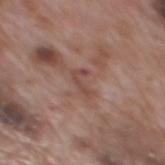Clinical impression: The lesion was photographed on a routine skin check and not biopsied; there is no pathology result. Clinical summary: The lesion is on the mid back. A male patient aged around 70. The total-body-photography lesion software estimated an average lesion color of about L≈46 a*≈21 b*≈23 (CIELAB) and about 7 CIELAB-L* units darker than the surrounding skin. The analysis additionally found a border-irregularity rating of about 7/10, internal color variation of about 0 on a 0–10 scale, and peripheral color asymmetry of about 0. This image is a 15 mm lesion crop taken from a total-body photograph.